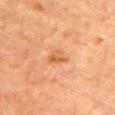follow-up = no biopsy performed (imaged during a skin exam) | body site = the front of the torso | illumination = cross-polarized | subject = female, aged around 70 | image = total-body-photography crop, ~15 mm field of view | TBP lesion metrics = a lesion area of about 4 mm² and an eccentricity of roughly 0.75; a lesion color around L≈52 a*≈23 b*≈37 in CIELAB and roughly 8 lightness units darker than nearby skin; a border-irregularity index near 3.5/10 and a color-variation rating of about 3.5/10; a nevus-likeness score of about 10/100 and a detector confidence of about 100 out of 100 that the crop contains a lesion | size = ≈3 mm.